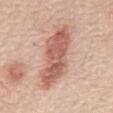{
  "biopsy_status": "not biopsied; imaged during a skin examination",
  "site": "mid back",
  "patient": {
    "sex": "male",
    "age_approx": 70
  },
  "lighting": "white-light",
  "image": {
    "source": "total-body photography crop",
    "field_of_view_mm": 15
  },
  "lesion_size": {
    "long_diameter_mm_approx": 9.0
  }
}